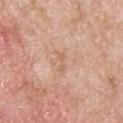Notes:
* follow-up: imaged on a skin check; not biopsied
* location: the upper back
* image-analysis metrics: two-axis asymmetry of about 0.4; a lesion–skin lightness drop of about 6 and a normalized border contrast of about 4.5; a classifier nevus-likeness of about 0/100 and a detector confidence of about 100 out of 100 that the crop contains a lesion
* patient: male, about 65 years old
* acquisition: ~15 mm crop, total-body skin-cancer survey
* lighting: white-light illumination